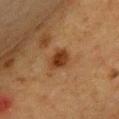Q: Was this lesion biopsied?
A: imaged on a skin check; not biopsied
Q: How was the tile lit?
A: cross-polarized
Q: What did automated image analysis measure?
A: an eccentricity of roughly 0.6 and a shape-asymmetry score of about 0.2 (0 = symmetric); an average lesion color of about L≈32 a*≈19 b*≈30 (CIELAB) and a normalized lesion–skin contrast near 9.5
Q: Who is the patient?
A: female, aged 53–57
Q: What is the lesion's diameter?
A: about 3 mm
Q: What is the anatomic site?
A: the chest
Q: What kind of image is this?
A: ~15 mm tile from a whole-body skin photo